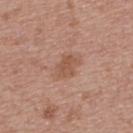Clinical impression:
The lesion was photographed on a routine skin check and not biopsied; there is no pathology result.
Context:
The patient is a female aged around 65. Automated image analysis of the tile measured a lesion color around L≈53 a*≈21 b*≈30 in CIELAB and roughly 8 lightness units darker than nearby skin. The analysis additionally found a border-irregularity index near 2.5/10, a within-lesion color-variation index near 1.5/10, and peripheral color asymmetry of about 0.5. Cropped from a whole-body photographic skin survey; the tile spans about 15 mm. The lesion is on the upper back. The tile uses white-light illumination.